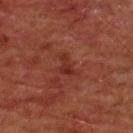Context:
On the chest. A 15 mm crop from a total-body photograph taken for skin-cancer surveillance. The patient is a male aged 58–62.